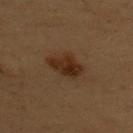Recorded during total-body skin imaging; not selected for excision or biopsy.
The patient is a female aged 58 to 62.
An algorithmic analysis of the crop reported a normalized border contrast of about 9.
Longest diameter approximately 4.5 mm.
Imaged with cross-polarized lighting.
From the back.
Cropped from a total-body skin-imaging series; the visible field is about 15 mm.